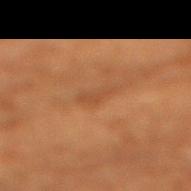No biopsy was performed on this lesion — it was imaged during a full skin examination and was not determined to be concerning.